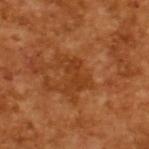acquisition = total-body-photography crop, ~15 mm field of view | subject = male, aged 63–67.